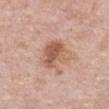No biopsy was performed on this lesion — it was imaged during a full skin examination and was not determined to be concerning.
The subject is a male aged 73 to 77.
This is a white-light tile.
The lesion is on the mid back.
A 15 mm close-up extracted from a 3D total-body photography capture.
Longest diameter approximately 5 mm.